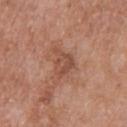The lesion was tiled from a total-body skin photograph and was not biopsied. The patient is a female aged 73–77. A 15 mm close-up tile from a total-body photography series done for melanoma screening. Located on the upper back. This is a white-light tile. The total-body-photography lesion software estimated an area of roughly 6.5 mm² and a shape-asymmetry score of about 0.5 (0 = symmetric). And it measured a lesion-detection confidence of about 100/100. Approximately 4 mm at its widest.